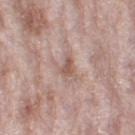* biopsy status: total-body-photography surveillance lesion; no biopsy
* imaging modality: ~15 mm crop, total-body skin-cancer survey
* lesion size: ≈2.5 mm
* body site: the right thigh
* patient: female, aged 68 to 72